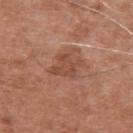This lesion was catalogued during total-body skin photography and was not selected for biopsy. Automated image analysis of the tile measured an outline eccentricity of about 0.6 (0 = round, 1 = elongated) and two-axis asymmetry of about 0.25. The software also gave an average lesion color of about L≈48 a*≈23 b*≈30 (CIELAB), roughly 8 lightness units darker than nearby skin, and a normalized lesion–skin contrast near 6.5. The software also gave an automated nevus-likeness rating near 0 out of 100 and lesion-presence confidence of about 100/100. The recorded lesion diameter is about 4 mm. The patient is a male aged 73 to 77. A lesion tile, about 15 mm wide, cut from a 3D total-body photograph. Located on the right upper arm.